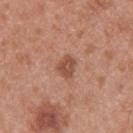- follow-up — imaged on a skin check; not biopsied
- anatomic site — the back
- patient — male, aged approximately 30
- imaging modality — ~15 mm tile from a whole-body skin photo